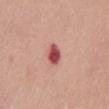Captured during whole-body skin photography for melanoma surveillance; the lesion was not biopsied. This image is a 15 mm lesion crop taken from a total-body photograph. Measured at roughly 2.5 mm in maximum diameter. Located on the chest. The lesion-visualizer software estimated an eccentricity of roughly 0.7 and a symmetry-axis asymmetry near 0.1. The software also gave a lesion color around L≈52 a*≈33 b*≈24 in CIELAB, roughly 17 lightness units darker than nearby skin, and a normalized border contrast of about 11. The analysis additionally found a within-lesion color-variation index near 4/10 and peripheral color asymmetry of about 1. A female patient approximately 65 years of age.